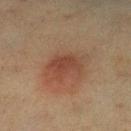image: ~15 mm crop, total-body skin-cancer survey; lighting: cross-polarized; size: ~4 mm (longest diameter); anatomic site: the left lower leg; patient: female, aged around 40.